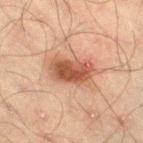Captured during whole-body skin photography for melanoma surveillance; the lesion was not biopsied. A 15 mm close-up tile from a total-body photography series done for melanoma screening. From the right thigh. An algorithmic analysis of the crop reported about 11 CIELAB-L* units darker than the surrounding skin and a lesion-to-skin contrast of about 9 (normalized; higher = more distinct). Captured under cross-polarized illumination. A male subject roughly 50 years of age.